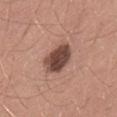Assessment:
This lesion was catalogued during total-body skin photography and was not selected for biopsy.
Context:
On the lower back. Cropped from a whole-body photographic skin survey; the tile spans about 15 mm. About 4.5 mm across. Captured under white-light illumination. A male subject, aged around 40.